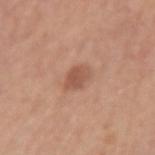{"biopsy_status": "not biopsied; imaged during a skin examination", "lighting": "white-light", "patient": {"sex": "male", "age_approx": 75}, "automated_metrics": {"area_mm2_approx": 4.5, "eccentricity": 0.7, "shape_asymmetry": 0.25, "cielab_L": 54, "cielab_a": 23, "cielab_b": 30, "vs_skin_darker_L": 9.0}, "site": "arm", "image": {"source": "total-body photography crop", "field_of_view_mm": 15}}